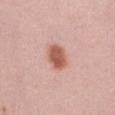Captured during whole-body skin photography for melanoma surveillance; the lesion was not biopsied. Located on the abdomen. Measured at roughly 3.5 mm in maximum diameter. An algorithmic analysis of the crop reported a footprint of about 7.5 mm² and an outline eccentricity of about 0.65 (0 = round, 1 = elongated). The analysis additionally found a nevus-likeness score of about 100/100. A lesion tile, about 15 mm wide, cut from a 3D total-body photograph. This is a white-light tile. A female subject, about 25 years old.